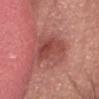Part of a total-body skin-imaging series; this lesion was reviewed on a skin check and was not flagged for biopsy. Cropped from a whole-body photographic skin survey; the tile spans about 15 mm. The patient is a male in their mid-40s. Measured at roughly 5 mm in maximum diameter. The lesion is located on the head or neck.